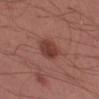Impression: Captured during whole-body skin photography for melanoma surveillance; the lesion was not biopsied. Background: A 15 mm close-up tile from a total-body photography series done for melanoma screening. Captured under white-light illumination. From the left upper arm. Approximately 3 mm at its widest. The subject is a male aged approximately 35.